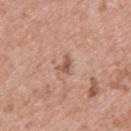Findings:
• follow-up: no biopsy performed (imaged during a skin exam)
• lesion size: about 2.5 mm
• site: the back
• patient: female, approximately 40 years of age
• imaging modality: total-body-photography crop, ~15 mm field of view